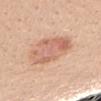Recorded during total-body skin imaging; not selected for excision or biopsy.
On the right forearm.
This is a white-light tile.
This image is a 15 mm lesion crop taken from a total-body photograph.
The total-body-photography lesion software estimated an area of roughly 19 mm² and two-axis asymmetry of about 0.15. It also reported a classifier nevus-likeness of about 55/100 and a lesion-detection confidence of about 100/100.
The subject is a female roughly 25 years of age.
About 5 mm across.